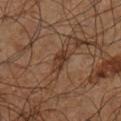Recorded during total-body skin imaging; not selected for excision or biopsy.
The lesion's longest dimension is about 3 mm.
Automated image analysis of the tile measured a normalized lesion–skin contrast near 8. The analysis additionally found border irregularity of about 2.5 on a 0–10 scale, a within-lesion color-variation index near 3/10, and radial color variation of about 1.
This is a cross-polarized tile.
A male subject, aged 58–62.
The lesion is located on the left lower leg.
A roughly 15 mm field-of-view crop from a total-body skin photograph.